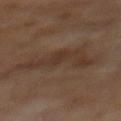Recorded during total-body skin imaging; not selected for excision or biopsy. A lesion tile, about 15 mm wide, cut from a 3D total-body photograph. From the mid back. A male patient aged 68 to 72.